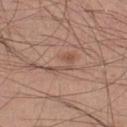Context: A male subject about 30 years old. A region of skin cropped from a whole-body photographic capture, roughly 15 mm wide. Located on the left lower leg.